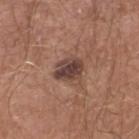This lesion was catalogued during total-body skin photography and was not selected for biopsy.
A 15 mm close-up extracted from a 3D total-body photography capture.
The lesion-visualizer software estimated an area of roughly 7 mm², an eccentricity of roughly 0.65, and a shape-asymmetry score of about 0.2 (0 = symmetric).
The tile uses white-light illumination.
The patient is a male aged 63–67.
Longest diameter approximately 3.5 mm.
The lesion is located on the right lower leg.